On the back; The total-body-photography lesion software estimated an area of roughly 20 mm², an eccentricity of roughly 0.9, and a shape-asymmetry score of about 0.15 (0 = symmetric). And it measured a lesion-to-skin contrast of about 8.5 (normalized; higher = more distinct). The analysis additionally found border irregularity of about 2.5 on a 0–10 scale, internal color variation of about 4 on a 0–10 scale, and a peripheral color-asymmetry measure near 1; the tile uses cross-polarized illumination; a male patient, about 30 years old; the lesion's longest dimension is about 7.5 mm; cropped from a total-body skin-imaging series; the visible field is about 15 mm:
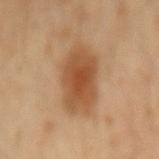Histopathology of the biopsied lesion showed an atypical melanocytic neoplasm (borderline).